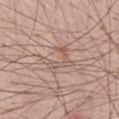No biopsy was performed on this lesion — it was imaged during a full skin examination and was not determined to be concerning. A male patient, in their mid-50s. The tile uses white-light illumination. Automated tile analysis of the lesion measured a footprint of about 5.5 mm² and two-axis asymmetry of about 0.7. And it measured a border-irregularity rating of about 10/10 and a peripheral color-asymmetry measure near 0. It also reported a classifier nevus-likeness of about 0/100. The recorded lesion diameter is about 4 mm. Cropped from a total-body skin-imaging series; the visible field is about 15 mm. Located on the front of the torso.